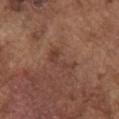This lesion was catalogued during total-body skin photography and was not selected for biopsy.